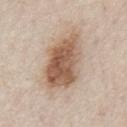Assessment: Recorded during total-body skin imaging; not selected for excision or biopsy. Context: This image is a 15 mm lesion crop taken from a total-body photograph. The subject is a male aged approximately 80. On the chest. Captured under white-light illumination.